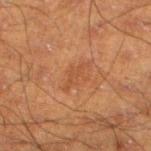Assessment:
Imaged during a routine full-body skin examination; the lesion was not biopsied and no histopathology is available.
Image and clinical context:
From the left lower leg. The subject is a male aged around 50. A 15 mm close-up tile from a total-body photography series done for melanoma screening.